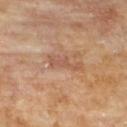Clinical impression: Imaged during a routine full-body skin examination; the lesion was not biopsied and no histopathology is available. Image and clinical context: From the upper back. About 4.5 mm across. A 15 mm close-up extracted from a 3D total-body photography capture. This is a cross-polarized tile. The subject is a male in their mid- to late 80s or older.